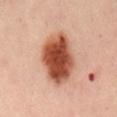workup = catalogued during a skin exam; not biopsied | patient = male, roughly 50 years of age | imaging modality = 15 mm crop, total-body photography | site = the mid back.